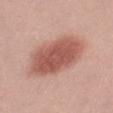Imaged during a routine full-body skin examination; the lesion was not biopsied and no histopathology is available.
A female subject, aged 18 to 22.
A close-up tile cropped from a whole-body skin photograph, about 15 mm across.
The lesion is on the leg.